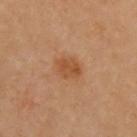The lesion was photographed on a routine skin check and not biopsied; there is no pathology result.
The subject is a female about 60 years old.
A 15 mm crop from a total-body photograph taken for skin-cancer surveillance.
The lesion is on the chest.
About 2.5 mm across.
This is a cross-polarized tile.
The lesion-visualizer software estimated a footprint of about 5.5 mm², an outline eccentricity of about 0.65 (0 = round, 1 = elongated), and a symmetry-axis asymmetry near 0.15. The analysis additionally found a color-variation rating of about 2.5/10 and radial color variation of about 1. The analysis additionally found a classifier nevus-likeness of about 50/100 and lesion-presence confidence of about 100/100.